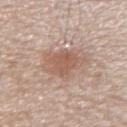  biopsy_status: not biopsied; imaged during a skin examination
  site: left forearm
  lesion_size:
    long_diameter_mm_approx: 5.0
  image:
    source: total-body photography crop
    field_of_view_mm: 15
  automated_metrics:
    area_mm2_approx: 12.0
    eccentricity: 0.75
    cielab_L: 57
    cielab_a: 19
    cielab_b: 27
    vs_skin_darker_L: 10.0
    border_irregularity_0_10: 4.0
    color_variation_0_10: 3.0
    peripheral_color_asymmetry: 1.0
  patient:
    sex: male
    age_approx: 40